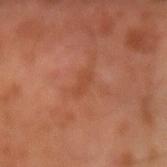Case summary:
* workup: no biopsy performed (imaged during a skin exam)
* subject: male, aged 63–67
* image source: 15 mm crop, total-body photography
* diameter: ≈3 mm
* illumination: cross-polarized illumination